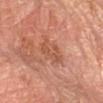workup=catalogued during a skin exam; not biopsied | illumination=cross-polarized | automated metrics=a border-irregularity index near 8/10, internal color variation of about 1.5 on a 0–10 scale, and peripheral color asymmetry of about 0.5 | image source=~15 mm tile from a whole-body skin photo | location=the left forearm | size=~3 mm (longest diameter) | patient=male, roughly 65 years of age.